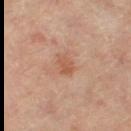notes: total-body-photography surveillance lesion; no biopsy.